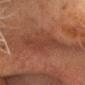Recorded during total-body skin imaging; not selected for excision or biopsy. A male patient, roughly 80 years of age. From the head or neck. A region of skin cropped from a whole-body photographic capture, roughly 15 mm wide.